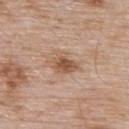Assessment:
Captured during whole-body skin photography for melanoma surveillance; the lesion was not biopsied.
Clinical summary:
From the back. The tile uses white-light illumination. A male patient aged 53–57. About 3.5 mm across. A 15 mm close-up tile from a total-body photography series done for melanoma screening.